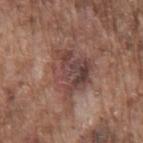This lesion was catalogued during total-body skin photography and was not selected for biopsy.